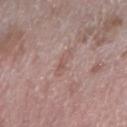Case summary:
• biopsy status: catalogued during a skin exam; not biopsied
• site: the right forearm
• image: total-body-photography crop, ~15 mm field of view
• patient: female, aged approximately 65